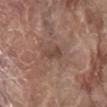image: ~15 mm crop, total-body skin-cancer survey | anatomic site: the mid back | patient: male, aged 78–82.